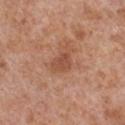Part of a total-body skin-imaging series; this lesion was reviewed on a skin check and was not flagged for biopsy.